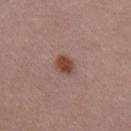Impression: Recorded during total-body skin imaging; not selected for excision or biopsy. Background: Approximately 2.5 mm at its widest. The lesion is on the right upper arm. This image is a 15 mm lesion crop taken from a total-body photograph. Imaged with white-light lighting. A female subject, approximately 30 years of age.